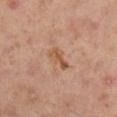Notes:
* biopsy status · no biopsy performed (imaged during a skin exam)
* lesion diameter · about 3 mm
* lighting · cross-polarized
* anatomic site · the right upper arm
* patient · male, aged 53–57
* TBP lesion metrics · a footprint of about 4.5 mm², an outline eccentricity of about 0.9 (0 = round, 1 = elongated), and a symmetry-axis asymmetry near 0.5; a lesion color around L≈54 a*≈21 b*≈34 in CIELAB, a lesion–skin lightness drop of about 9, and a normalized border contrast of about 7.5; a border-irregularity rating of about 5/10, internal color variation of about 2.5 on a 0–10 scale, and a peripheral color-asymmetry measure near 1; an automated nevus-likeness rating near 0 out of 100
* image · ~15 mm tile from a whole-body skin photo